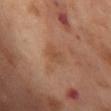Captured under cross-polarized illumination.
Longest diameter approximately 2.5 mm.
A close-up tile cropped from a whole-body skin photograph, about 15 mm across.
Automated image analysis of the tile measured a mean CIELAB color near L≈50 a*≈21 b*≈33, about 6 CIELAB-L* units darker than the surrounding skin, and a normalized lesion–skin contrast near 5. And it measured lesion-presence confidence of about 100/100.
A female subject, in their mid-50s.
The lesion is on the abdomen.